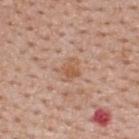Assessment: Captured during whole-body skin photography for melanoma surveillance; the lesion was not biopsied. Acquisition and patient details: The patient is a male approximately 40 years of age. The recorded lesion diameter is about 3 mm. On the upper back. Imaged with white-light lighting. Automated image analysis of the tile measured a lesion color around L≈57 a*≈21 b*≈32 in CIELAB and a lesion–skin lightness drop of about 7. And it measured a color-variation rating of about 3/10 and a peripheral color-asymmetry measure near 1. The software also gave a detector confidence of about 100 out of 100 that the crop contains a lesion. Cropped from a whole-body photographic skin survey; the tile spans about 15 mm.